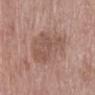- biopsy status — no biopsy performed (imaged during a skin exam)
- subject — female, roughly 75 years of age
- size — ≈7 mm
- site — the abdomen
- tile lighting — white-light illumination
- image — ~15 mm crop, total-body skin-cancer survey
- automated metrics — an average lesion color of about L≈53 a*≈18 b*≈25 (CIELAB), about 8 CIELAB-L* units darker than the surrounding skin, and a normalized border contrast of about 6; a border-irregularity index near 2.5/10 and a within-lesion color-variation index near 3.5/10